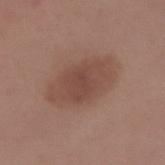Context:
The total-body-photography lesion software estimated an area of roughly 21 mm² and an eccentricity of roughly 0.75. The software also gave a mean CIELAB color near L≈46 a*≈20 b*≈24 and a lesion–skin lightness drop of about 8. The analysis additionally found border irregularity of about 1.5 on a 0–10 scale, internal color variation of about 2.5 on a 0–10 scale, and peripheral color asymmetry of about 0.5. Captured under white-light illumination. Measured at roughly 6.5 mm in maximum diameter. The subject is a female aged 23 to 27. This image is a 15 mm lesion crop taken from a total-body photograph. Located on the right forearm.